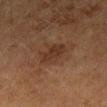biopsy status: imaged on a skin check; not biopsied | location: the left forearm | patient: female, in their mid-50s | imaging modality: ~15 mm tile from a whole-body skin photo | automated lesion analysis: a lesion area of about 7.5 mm², an eccentricity of roughly 0.65, and a symmetry-axis asymmetry near 0.2; border irregularity of about 2 on a 0–10 scale and a color-variation rating of about 2.5/10; an automated nevus-likeness rating near 0 out of 100 and a detector confidence of about 100 out of 100 that the crop contains a lesion | illumination: cross-polarized illumination | lesion diameter: ≈3.5 mm.